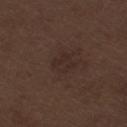The lesion was photographed on a routine skin check and not biopsied; there is no pathology result. On the right thigh. The subject is a male in their 70s. A close-up tile cropped from a whole-body skin photograph, about 15 mm across. The tile uses white-light illumination. Approximately 2.5 mm at its widest.